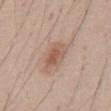Assessment:
This lesion was catalogued during total-body skin photography and was not selected for biopsy.